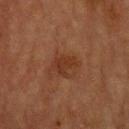image = total-body-photography crop, ~15 mm field of view | image-analysis metrics = border irregularity of about 3.5 on a 0–10 scale and a peripheral color-asymmetry measure near 0.5; a classifier nevus-likeness of about 5/100 and a lesion-detection confidence of about 100/100 | body site = the head or neck | subject = male, roughly 60 years of age.